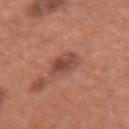Q: What are the patient's age and sex?
A: female, about 65 years old
Q: What is the imaging modality?
A: ~15 mm tile from a whole-body skin photo
Q: What did automated image analysis measure?
A: an area of roughly 7 mm² and a shape-asymmetry score of about 0.15 (0 = symmetric); about 10 CIELAB-L* units darker than the surrounding skin and a normalized border contrast of about 7.5; a peripheral color-asymmetry measure near 1.5
Q: How large is the lesion?
A: about 3 mm
Q: Lesion location?
A: the right forearm